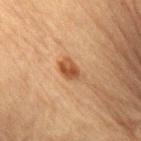Imaged during a routine full-body skin examination; the lesion was not biopsied and no histopathology is available. From the lower back. Imaged with cross-polarized lighting. A 15 mm crop from a total-body photograph taken for skin-cancer surveillance. The subject is a male aged approximately 85 or older. The lesion's longest dimension is about 3 mm.